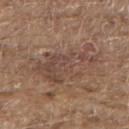Q: Was this lesion biopsied?
A: no biopsy performed (imaged during a skin exam)
Q: What is the lesion's diameter?
A: ≈8 mm
Q: Who is the patient?
A: male, aged around 80
Q: What did automated image analysis measure?
A: internal color variation of about 3 on a 0–10 scale; an automated nevus-likeness rating near 0 out of 100 and lesion-presence confidence of about 55/100
Q: Where on the body is the lesion?
A: the upper back
Q: What is the imaging modality?
A: total-body-photography crop, ~15 mm field of view